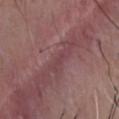This lesion was catalogued during total-body skin photography and was not selected for biopsy. The lesion is on the front of the torso. A male subject about 50 years old. Cropped from a whole-body photographic skin survey; the tile spans about 15 mm. Automated image analysis of the tile measured a normalized lesion–skin contrast near 4. About 1.5 mm across. This is a white-light tile.